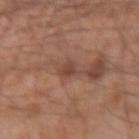follow-up: total-body-photography surveillance lesion; no biopsy | patient: male, aged 28–32 | site: the arm | imaging modality: 15 mm crop, total-body photography.